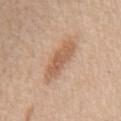• biopsy status — no biopsy performed (imaged during a skin exam)
• anatomic site — the mid back
• diameter — ~5.5 mm (longest diameter)
• subject — male, aged approximately 60
• image source — ~15 mm crop, total-body skin-cancer survey